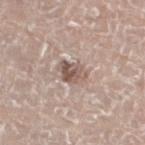biopsy_status: not biopsied; imaged during a skin examination
patient:
  sex: male
  age_approx: 75
automated_metrics:
  cielab_L: 55
  cielab_a: 16
  cielab_b: 23
  border_irregularity_0_10: 2.5
  color_variation_0_10: 6.5
  peripheral_color_asymmetry: 2.5
site: leg
lighting: white-light
image:
  source: total-body photography crop
  field_of_view_mm: 15